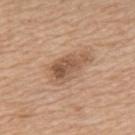This lesion was catalogued during total-body skin photography and was not selected for biopsy.
Cropped from a whole-body photographic skin survey; the tile spans about 15 mm.
An algorithmic analysis of the crop reported a lesion area of about 11 mm², a shape eccentricity near 0.85, and a symmetry-axis asymmetry near 0.35. The software also gave an average lesion color of about L≈55 a*≈18 b*≈31 (CIELAB) and roughly 11 lightness units darker than nearby skin. It also reported radial color variation of about 2. The analysis additionally found a classifier nevus-likeness of about 40/100 and a lesion-detection confidence of about 100/100.
This is a white-light tile.
From the upper back.
Approximately 5.5 mm at its widest.
A male subject, aged around 65.